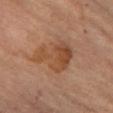Assessment: This lesion was catalogued during total-body skin photography and was not selected for biopsy.